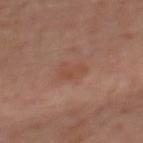notes = total-body-photography surveillance lesion; no biopsy.